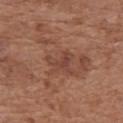Q: Was a biopsy performed?
A: imaged on a skin check; not biopsied
Q: What kind of image is this?
A: total-body-photography crop, ~15 mm field of view
Q: What is the anatomic site?
A: the back
Q: Patient demographics?
A: female, aged around 75